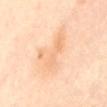Clinical impression: The lesion was tiled from a total-body skin photograph and was not biopsied. Background: A female subject, aged approximately 40. On the back. Measured at roughly 6.5 mm in maximum diameter. A close-up tile cropped from a whole-body skin photograph, about 15 mm across. This is a cross-polarized tile.